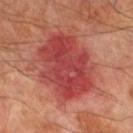The lesion was tiled from a total-body skin photograph and was not biopsied.
The patient is a male aged approximately 70.
A close-up tile cropped from a whole-body skin photograph, about 15 mm across.
The lesion is on the left lower leg.